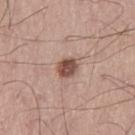notes: no biopsy performed (imaged during a skin exam)
image source: ~15 mm crop, total-body skin-cancer survey
size: ~2.5 mm (longest diameter)
patient: male, aged 58 to 62
location: the left thigh